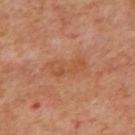Findings:
- notes — total-body-photography surveillance lesion; no biopsy
- acquisition — ~15 mm crop, total-body skin-cancer survey
- site — the mid back
- subject — in their mid-60s